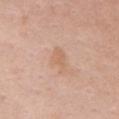notes — total-body-photography surveillance lesion; no biopsy | site — the front of the torso | patient — female, in their mid- to late 60s | image source — 15 mm crop, total-body photography | illumination — white-light.